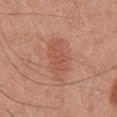Recorded during total-body skin imaging; not selected for excision or biopsy.